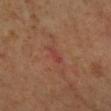A female patient, about 65 years old. On the left forearm. Cropped from a whole-body photographic skin survey; the tile spans about 15 mm.